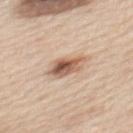<record>
<biopsy_status>not biopsied; imaged during a skin examination</biopsy_status>
<image>
  <source>total-body photography crop</source>
  <field_of_view_mm>15</field_of_view_mm>
</image>
<site>mid back</site>
<lighting>white-light</lighting>
<lesion_size>
  <long_diameter_mm_approx>5.0</long_diameter_mm_approx>
</lesion_size>
<patient>
  <sex>male</sex>
  <age_approx>60</age_approx>
</patient>
</record>